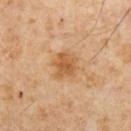| key | value |
|---|---|
| follow-up | no biopsy performed (imaged during a skin exam) |
| illumination | cross-polarized illumination |
| image | ~15 mm tile from a whole-body skin photo |
| diameter | about 3 mm |
| subject | male, aged around 65 |
| image-analysis metrics | a footprint of about 7 mm², an outline eccentricity of about 0.45 (0 = round, 1 = elongated), and a shape-asymmetry score of about 0.3 (0 = symmetric) |